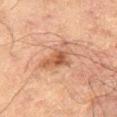biopsy status: imaged on a skin check; not biopsied
image: ~15 mm crop, total-body skin-cancer survey
automated lesion analysis: an area of roughly 9 mm² and a shape-asymmetry score of about 0.4 (0 = symmetric); a border-irregularity index near 4.5/10, a color-variation rating of about 4.5/10, and a peripheral color-asymmetry measure near 1; lesion-presence confidence of about 100/100
tile lighting: cross-polarized
body site: the leg
diameter: ≈4.5 mm
patient: male, approximately 70 years of age